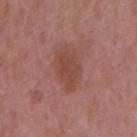Part of a total-body skin-imaging series; this lesion was reviewed on a skin check and was not flagged for biopsy. The lesion is on the mid back. A male patient in their mid-50s. Automated tile analysis of the lesion measured a footprint of about 11 mm², a shape eccentricity near 0.85, and a shape-asymmetry score of about 0.2 (0 = symmetric). The analysis additionally found an average lesion color of about L≈46 a*≈25 b*≈26 (CIELAB), a lesion–skin lightness drop of about 7, and a normalized lesion–skin contrast near 6. A close-up tile cropped from a whole-body skin photograph, about 15 mm across.